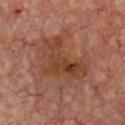| feature | finding |
|---|---|
| biopsy status | catalogued during a skin exam; not biopsied |
| size | ~6.5 mm (longest diameter) |
| patient | in their mid- to late 60s |
| image | total-body-photography crop, ~15 mm field of view |
| illumination | cross-polarized illumination |
| location | the front of the torso |